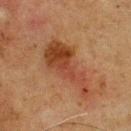Recorded during total-body skin imaging; not selected for excision or biopsy.
A male subject, approximately 75 years of age.
The recorded lesion diameter is about 8 mm.
Captured under cross-polarized illumination.
Cropped from a whole-body photographic skin survey; the tile spans about 15 mm.
Located on the chest.
Automated tile analysis of the lesion measured roughly 9 lightness units darker than nearby skin and a lesion-to-skin contrast of about 8 (normalized; higher = more distinct). The analysis additionally found a classifier nevus-likeness of about 40/100 and a detector confidence of about 100 out of 100 that the crop contains a lesion.